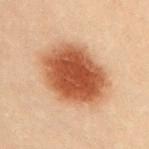This lesion was catalogued during total-body skin photography and was not selected for biopsy.
The tile uses cross-polarized illumination.
The subject is a female in their 20s.
The lesion is located on the front of the torso.
Automated image analysis of the tile measured a footprint of about 32 mm², a shape eccentricity near 0.7, and a shape-asymmetry score of about 0.15 (0 = symmetric). And it measured a mean CIELAB color near L≈41 a*≈22 b*≈30 and a lesion–skin lightness drop of about 15. The software also gave a border-irregularity index near 1.5/10 and a within-lesion color-variation index near 5/10.
Cropped from a whole-body photographic skin survey; the tile spans about 15 mm.